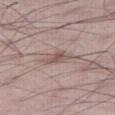Clinical impression: Captured during whole-body skin photography for melanoma surveillance; the lesion was not biopsied. Context: The lesion-visualizer software estimated a nevus-likeness score of about 0/100 and a lesion-detection confidence of about 70/100. The patient is a male about 70 years old. A 15 mm close-up extracted from a 3D total-body photography capture. The lesion is located on the right thigh.